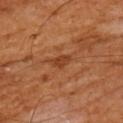Clinical summary:
A 15 mm crop from a total-body photograph taken for skin-cancer surveillance. The lesion is located on the upper back. An algorithmic analysis of the crop reported an average lesion color of about L≈39 a*≈25 b*≈35 (CIELAB) and a lesion–skin lightness drop of about 8. A male subject, roughly 65 years of age.